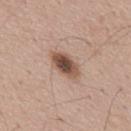Impression:
Part of a total-body skin-imaging series; this lesion was reviewed on a skin check and was not flagged for biopsy.
Image and clinical context:
Captured under white-light illumination. A male subject roughly 55 years of age. A 15 mm close-up extracted from a 3D total-body photography capture. The recorded lesion diameter is about 4.5 mm.